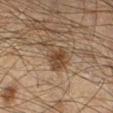No biopsy was performed on this lesion — it was imaged during a full skin examination and was not determined to be concerning. The lesion's longest dimension is about 4.5 mm. This image is a 15 mm lesion crop taken from a total-body photograph. On the right lower leg. Automated tile analysis of the lesion measured an area of roughly 7.5 mm² and two-axis asymmetry of about 0.55. It also reported a lesion color around L≈33 a*≈13 b*≈24 in CIELAB, a lesion–skin lightness drop of about 8, and a normalized lesion–skin contrast near 8.5. The software also gave a within-lesion color-variation index near 2.5/10 and peripheral color asymmetry of about 1. This is a cross-polarized tile. A male patient, roughly 50 years of age.